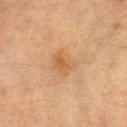The lesion was tiled from a total-body skin photograph and was not biopsied.
The lesion is on the left thigh.
Imaged with cross-polarized lighting.
A female patient aged 53 to 57.
Longest diameter approximately 3 mm.
A region of skin cropped from a whole-body photographic capture, roughly 15 mm wide.